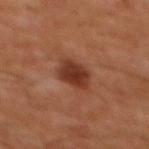Findings:
- follow-up — catalogued during a skin exam; not biopsied
- acquisition — ~15 mm tile from a whole-body skin photo
- subject — male, roughly 60 years of age
- location — the chest
- lesion diameter — about 3.5 mm